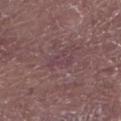{"biopsy_status": "not biopsied; imaged during a skin examination", "patient": {"sex": "male", "age_approx": 80}, "lighting": "white-light", "lesion_size": {"long_diameter_mm_approx": 3.0}, "image": {"source": "total-body photography crop", "field_of_view_mm": 15}, "site": "left lower leg"}